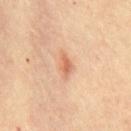The lesion was photographed on a routine skin check and not biopsied; there is no pathology result. A lesion tile, about 15 mm wide, cut from a 3D total-body photograph. Longest diameter approximately 3 mm. The patient is a female roughly 45 years of age. The lesion is on the chest. Automated image analysis of the tile measured a lesion area of about 3.5 mm² and a shape-asymmetry score of about 0.3 (0 = symmetric).